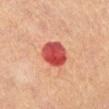Imaged during a routine full-body skin examination; the lesion was not biopsied and no histopathology is available.
The recorded lesion diameter is about 4 mm.
A 15 mm close-up extracted from a 3D total-body photography capture.
A female subject, aged around 60.
The lesion is located on the leg.
The total-body-photography lesion software estimated a mean CIELAB color near L≈45 a*≈36 b*≈29, roughly 16 lightness units darker than nearby skin, and a normalized border contrast of about 11.5. And it measured a border-irregularity rating of about 1/10, a within-lesion color-variation index near 6/10, and a peripheral color-asymmetry measure near 2. The software also gave a classifier nevus-likeness of about 0/100.